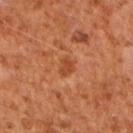workup: imaged on a skin check; not biopsied
imaging modality: ~15 mm crop, total-body skin-cancer survey
location: the right forearm
image-analysis metrics: a shape eccentricity near 0.8; a mean CIELAB color near L≈43 a*≈27 b*≈37, about 8 CIELAB-L* units darker than the surrounding skin, and a lesion-to-skin contrast of about 6.5 (normalized; higher = more distinct); a nevus-likeness score of about 5/100
patient: male, aged 58–62